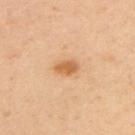follow-up=catalogued during a skin exam; not biopsied | image=total-body-photography crop, ~15 mm field of view | illumination=cross-polarized illumination | patient=male, aged 43–47 | diameter=≈3 mm | site=the upper back.